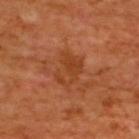Captured during whole-body skin photography for melanoma surveillance; the lesion was not biopsied. A male patient, in their mid- to late 60s. An algorithmic analysis of the crop reported a mean CIELAB color near L≈41 a*≈27 b*≈38 and roughly 7 lightness units darker than nearby skin. The software also gave a classifier nevus-likeness of about 0/100. The lesion's longest dimension is about 4 mm. On the upper back. This is a cross-polarized tile. A close-up tile cropped from a whole-body skin photograph, about 15 mm across.